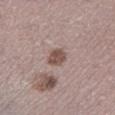Notes:
– follow-up · imaged on a skin check; not biopsied
– imaging modality · ~15 mm crop, total-body skin-cancer survey
– lighting · white-light illumination
– patient · male, aged around 70
– diameter · ≈2.5 mm
– automated metrics · about 12 CIELAB-L* units darker than the surrounding skin and a normalized border contrast of about 8.5
– site · the left lower leg